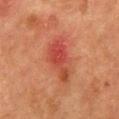No biopsy was performed on this lesion — it was imaged during a full skin examination and was not determined to be concerning.
A female patient, aged approximately 50.
A close-up tile cropped from a whole-body skin photograph, about 15 mm across.
On the chest.
About 6 mm across.